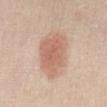Assessment: Captured during whole-body skin photography for melanoma surveillance; the lesion was not biopsied. Acquisition and patient details: About 6 mm across. From the abdomen. A female subject, in their 40s. A roughly 15 mm field-of-view crop from a total-body skin photograph.